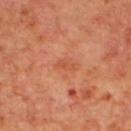A male subject aged 68–72.
Located on the upper back.
The total-body-photography lesion software estimated an average lesion color of about L≈52 a*≈29 b*≈37 (CIELAB), a lesion–skin lightness drop of about 6, and a normalized border contrast of about 5.
Measured at roughly 2.5 mm in maximum diameter.
Cropped from a whole-body photographic skin survey; the tile spans about 15 mm.